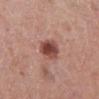notes — imaged on a skin check; not biopsied | tile lighting — white-light | body site — the right lower leg | subject — female, aged around 65 | lesion diameter — ~3.5 mm (longest diameter) | image source — ~15 mm tile from a whole-body skin photo.